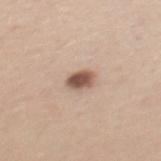Clinical impression:
The lesion was photographed on a routine skin check and not biopsied; there is no pathology result.
Clinical summary:
A 15 mm close-up tile from a total-body photography series done for melanoma screening. The lesion is located on the mid back. The tile uses white-light illumination. A female patient, aged 38–42. Approximately 3 mm at its widest.